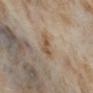Findings:
* anatomic site · the left lower leg
* diameter · ~3 mm (longest diameter)
* illumination · cross-polarized
* patient · female, in their mid- to late 50s
* image source · total-body-photography crop, ~15 mm field of view